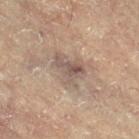<lesion>
  <biopsy_status>not biopsied; imaged during a skin examination</biopsy_status>
  <automated_metrics>
    <border_irregularity_0_10>5.5</border_irregularity_0_10>
    <color_variation_0_10>5.5</color_variation_0_10>
    <peripheral_color_asymmetry>2.0</peripheral_color_asymmetry>
  </automated_metrics>
  <site>left leg</site>
  <image>
    <source>total-body photography crop</source>
    <field_of_view_mm>15</field_of_view_mm>
  </image>
  <patient>
    <sex>female</sex>
    <age_approx>80</age_approx>
  </patient>
  <lighting>cross-polarized</lighting>
  <lesion_size>
    <long_diameter_mm_approx>4.5</long_diameter_mm_approx>
  </lesion_size>
</lesion>